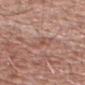workup=catalogued during a skin exam; not biopsied
patient=male, roughly 65 years of age
image source=total-body-photography crop, ~15 mm field of view
illumination=white-light
diameter=≈2.5 mm
site=the head or neck